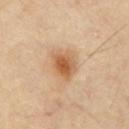follow-up: total-body-photography surveillance lesion; no biopsy
image source: ~15 mm crop, total-body skin-cancer survey
size: about 4 mm
location: the chest
illumination: cross-polarized illumination
image-analysis metrics: a lesion area of about 9 mm², an outline eccentricity of about 0.65 (0 = round, 1 = elongated), and two-axis asymmetry of about 0.25; an average lesion color of about L≈61 a*≈21 b*≈39 (CIELAB), about 12 CIELAB-L* units darker than the surrounding skin, and a lesion-to-skin contrast of about 8 (normalized; higher = more distinct)